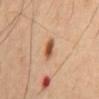- image-analysis metrics — a lesion area of about 4 mm², an eccentricity of roughly 0.8, and a shape-asymmetry score of about 0.2 (0 = symmetric); a mean CIELAB color near L≈51 a*≈21 b*≈34, about 13 CIELAB-L* units darker than the surrounding skin, and a normalized border contrast of about 9.5; border irregularity of about 1.5 on a 0–10 scale, a color-variation rating of about 4.5/10, and a peripheral color-asymmetry measure near 1.5; an automated nevus-likeness rating near 100 out of 100 and a detector confidence of about 100 out of 100 that the crop contains a lesion
- location — the mid back
- subject — male, about 45 years old
- tile lighting — cross-polarized illumination
- image source — 15 mm crop, total-body photography
- size — ~2.5 mm (longest diameter)